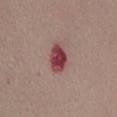Recorded during total-body skin imaging; not selected for excision or biopsy.
The tile uses white-light illumination.
The patient is a female aged 48–52.
About 3.5 mm across.
From the abdomen.
A 15 mm close-up tile from a total-body photography series done for melanoma screening.